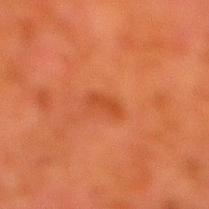<tbp_lesion>
  <biopsy_status>not biopsied; imaged during a skin examination</biopsy_status>
  <image>
    <source>total-body photography crop</source>
    <field_of_view_mm>15</field_of_view_mm>
  </image>
  <lesion_size>
    <long_diameter_mm_approx>2.5</long_diameter_mm_approx>
  </lesion_size>
  <automated_metrics>
    <cielab_L>38</cielab_L>
    <cielab_a>29</cielab_a>
    <cielab_b>36</cielab_b>
    <vs_skin_darker_L>6.0</vs_skin_darker_L>
    <vs_skin_contrast_norm>5.5</vs_skin_contrast_norm>
    <border_irregularity_0_10>3.5</border_irregularity_0_10>
    <color_variation_0_10>0.5</color_variation_0_10>
    <peripheral_color_asymmetry>0.0</peripheral_color_asymmetry>
    <lesion_detection_confidence_0_100>100</lesion_detection_confidence_0_100>
  </automated_metrics>
  <patient>
    <sex>male</sex>
    <age_approx>80</age_approx>
  </patient>
  <site>leg</site>
  <lighting>cross-polarized</lighting>
</tbp_lesion>